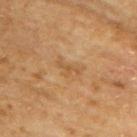Q: Was a biopsy performed?
A: imaged on a skin check; not biopsied
Q: What is the anatomic site?
A: the back
Q: Who is the patient?
A: male, approximately 65 years of age
Q: What kind of image is this?
A: 15 mm crop, total-body photography